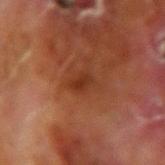Recorded during total-body skin imaging; not selected for excision or biopsy. The lesion's longest dimension is about 2.5 mm. A region of skin cropped from a whole-body photographic capture, roughly 15 mm wide. Captured under cross-polarized illumination. A male patient, about 70 years old. The lesion is on the arm.